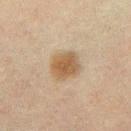– image · ~15 mm tile from a whole-body skin photo
– patient · male, approximately 50 years of age
– automated metrics · an average lesion color of about L≈43 a*≈12 b*≈28 (CIELAB) and a lesion-to-skin contrast of about 8.5 (normalized; higher = more distinct); a classifier nevus-likeness of about 95/100
– illumination · cross-polarized
– size · about 3.5 mm
– anatomic site · the abdomen